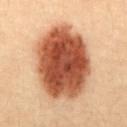The lesion was tiled from a total-body skin photograph and was not biopsied.
A female patient aged 18 to 22.
About 8.5 mm across.
The total-body-photography lesion software estimated a mean CIELAB color near L≈40 a*≈22 b*≈29, a lesion–skin lightness drop of about 18, and a normalized lesion–skin contrast near 13.5. The software also gave border irregularity of about 1.5 on a 0–10 scale, internal color variation of about 6 on a 0–10 scale, and peripheral color asymmetry of about 1.5. And it measured a classifier nevus-likeness of about 100/100 and lesion-presence confidence of about 100/100.
From the abdomen.
A lesion tile, about 15 mm wide, cut from a 3D total-body photograph.